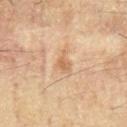Longest diameter approximately 2.5 mm.
Automated image analysis of the tile measured a footprint of about 3.5 mm² and two-axis asymmetry of about 0.45. The analysis additionally found a border-irregularity rating of about 4.5/10, a within-lesion color-variation index near 1.5/10, and peripheral color asymmetry of about 0.5.
Captured under cross-polarized illumination.
A 15 mm close-up extracted from a 3D total-body photography capture.
A male subject, aged 58 to 62.